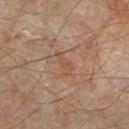Clinical impression:
Recorded during total-body skin imaging; not selected for excision or biopsy.
Background:
Imaged with cross-polarized lighting. Cropped from a total-body skin-imaging series; the visible field is about 15 mm. The patient is roughly 55 years of age. About 3 mm across. On the right lower leg.